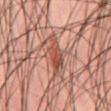Q: Was a biopsy performed?
A: imaged on a skin check; not biopsied
Q: What did automated image analysis measure?
A: a lesion area of about 7.5 mm² and two-axis asymmetry of about 0.35
Q: Lesion size?
A: ~5.5 mm (longest diameter)
Q: What lighting was used for the tile?
A: cross-polarized illumination
Q: What is the anatomic site?
A: the front of the torso
Q: Patient demographics?
A: male, aged 43–47
Q: What is the imaging modality?
A: ~15 mm crop, total-body skin-cancer survey